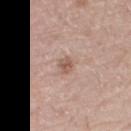Assessment:
Captured during whole-body skin photography for melanoma surveillance; the lesion was not biopsied.
Acquisition and patient details:
An algorithmic analysis of the crop reported a footprint of about 3 mm² and a shape eccentricity near 0.75. The analysis additionally found a mean CIELAB color near L≈56 a*≈19 b*≈26 and a lesion–skin lightness drop of about 10. The software also gave a nevus-likeness score of about 0/100 and a lesion-detection confidence of about 100/100. Cropped from a total-body skin-imaging series; the visible field is about 15 mm. A female subject, roughly 70 years of age. From the right thigh.